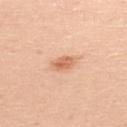{"site": "back", "automated_metrics": {"cielab_L": 66, "cielab_a": 25, "cielab_b": 36, "vs_skin_contrast_norm": 7.0, "nevus_likeness_0_100": 80, "lesion_detection_confidence_0_100": 100}, "lighting": "white-light", "lesion_size": {"long_diameter_mm_approx": 2.5}, "patient": {"sex": "male", "age_approx": 45}, "image": {"source": "total-body photography crop", "field_of_view_mm": 15}}